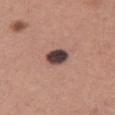site: leg
lighting: white-light
image:
  source: total-body photography crop
  field_of_view_mm: 15
patient:
  sex: female
  age_approx: 30
lesion_size:
  long_diameter_mm_approx: 3.0
automated_metrics:
  vs_skin_darker_L: 21.0
diagnosis:
  histopathology: dysplastic (Clark) nevus
  malignancy: benign
  taxonomic_path:
    - Benign
    - Benign melanocytic proliferations
    - Nevus
    - Nevus, Atypical, Dysplastic, or Clark